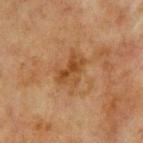Part of a total-body skin-imaging series; this lesion was reviewed on a skin check and was not flagged for biopsy. Imaged with cross-polarized lighting. A 15 mm close-up tile from a total-body photography series done for melanoma screening. The lesion is on the chest. The patient is a male in their mid-60s. The lesion's longest dimension is about 4.5 mm. The total-body-photography lesion software estimated a lesion color around L≈38 a*≈18 b*≈32 in CIELAB, roughly 7 lightness units darker than nearby skin, and a lesion-to-skin contrast of about 7 (normalized; higher = more distinct). The analysis additionally found a border-irregularity index near 6/10, a within-lesion color-variation index near 4.5/10, and a peripheral color-asymmetry measure near 1.5.